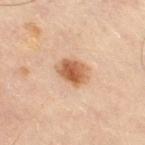Impression:
This lesion was catalogued during total-body skin photography and was not selected for biopsy.
Image and clinical context:
Captured under cross-polarized illumination. From the right thigh. The recorded lesion diameter is about 4 mm. A 15 mm close-up tile from a total-body photography series done for melanoma screening. The subject is approximately 55 years of age. Automated image analysis of the tile measured a mean CIELAB color near L≈59 a*≈23 b*≈36, roughly 14 lightness units darker than nearby skin, and a normalized border contrast of about 9.5. It also reported internal color variation of about 6 on a 0–10 scale and peripheral color asymmetry of about 2.